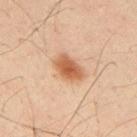size — about 3.5 mm
location — the upper back
imaging modality — total-body-photography crop, ~15 mm field of view
illumination — cross-polarized illumination
patient — male, approximately 50 years of age
automated lesion analysis — a lesion color around L≈53 a*≈22 b*≈34 in CIELAB, a lesion–skin lightness drop of about 12, and a lesion-to-skin contrast of about 9 (normalized; higher = more distinct); border irregularity of about 1.5 on a 0–10 scale, a color-variation rating of about 3.5/10, and peripheral color asymmetry of about 1; a nevus-likeness score of about 100/100 and a lesion-detection confidence of about 100/100